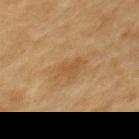The lesion was photographed on a routine skin check and not biopsied; there is no pathology result.
Imaged with cross-polarized lighting.
A lesion tile, about 15 mm wide, cut from a 3D total-body photograph.
The subject is a female aged around 60.
The recorded lesion diameter is about 3.5 mm.
The lesion is located on the upper back.